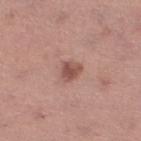Clinical impression:
Recorded during total-body skin imaging; not selected for excision or biopsy.
Acquisition and patient details:
A female patient, aged approximately 55. The lesion's longest dimension is about 2.5 mm. A lesion tile, about 15 mm wide, cut from a 3D total-body photograph. Captured under white-light illumination. Located on the left lower leg.